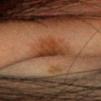Imaged during a routine full-body skin examination; the lesion was not biopsied and no histopathology is available. A roughly 15 mm field-of-view crop from a total-body skin photograph. Approximately 4.5 mm at its widest. A female patient, approximately 30 years of age. The lesion is located on the head or neck. The tile uses cross-polarized illumination.